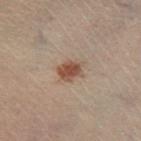* follow-up · imaged on a skin check; not biopsied
* tile lighting · cross-polarized illumination
* lesion diameter · about 3 mm
* TBP lesion metrics · a lesion area of about 6 mm²; a mean CIELAB color near L≈39 a*≈16 b*≈23, about 9 CIELAB-L* units darker than the surrounding skin, and a normalized border contrast of about 8.5; a nevus-likeness score of about 95/100 and lesion-presence confidence of about 100/100
* location · the leg
* image · ~15 mm tile from a whole-body skin photo
* patient · male, aged around 55